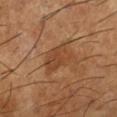biopsy status=catalogued during a skin exam; not biopsied
image-analysis metrics=an average lesion color of about L≈44 a*≈22 b*≈34 (CIELAB)
tile lighting=cross-polarized illumination
subject=male, in their mid-60s
imaging modality=~15 mm crop, total-body skin-cancer survey
diameter=about 4.5 mm
anatomic site=the left lower leg